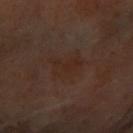{
  "lighting": "cross-polarized",
  "site": "left arm",
  "automated_metrics": {
    "vs_skin_darker_L": 3.0,
    "vs_skin_contrast_norm": 4.5,
    "border_irregularity_0_10": 4.0,
    "color_variation_0_10": 2.5,
    "peripheral_color_asymmetry": 1.0
  },
  "image": {
    "source": "total-body photography crop",
    "field_of_view_mm": 15
  },
  "patient": {
    "sex": "female",
    "age_approx": 60
  }
}